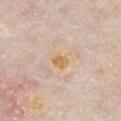{
  "biopsy_status": "not biopsied; imaged during a skin examination",
  "patient": {
    "sex": "male",
    "age_approx": 75
  },
  "image": {
    "source": "total-body photography crop",
    "field_of_view_mm": 15
  },
  "site": "front of the torso"
}